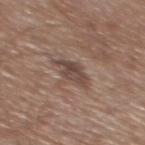notes: total-body-photography surveillance lesion; no biopsy | lesion size: ~4 mm (longest diameter) | location: the mid back | image: ~15 mm tile from a whole-body skin photo | automated lesion analysis: a footprint of about 6.5 mm², an eccentricity of roughly 0.8, and a shape-asymmetry score of about 0.25 (0 = symmetric); a lesion color around L≈45 a*≈16 b*≈22 in CIELAB and a normalized lesion–skin contrast near 8; border irregularity of about 3 on a 0–10 scale and radial color variation of about 1.5 | subject: male, aged around 65.